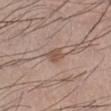Impression:
The lesion was photographed on a routine skin check and not biopsied; there is no pathology result.
Context:
This is a white-light tile. Cropped from a whole-body photographic skin survey; the tile spans about 15 mm. The subject is a male roughly 55 years of age. Longest diameter approximately 2.5 mm.